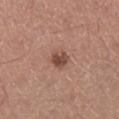<tbp_lesion>
<biopsy_status>not biopsied; imaged during a skin examination</biopsy_status>
<automated_metrics>
  <area_mm2_approx>4.5</area_mm2_approx>
  <shape_asymmetry>0.2</shape_asymmetry>
  <cielab_L>47</cielab_L>
  <cielab_a>21</cielab_a>
  <cielab_b>25</cielab_b>
  <vs_skin_darker_L>12.0</vs_skin_darker_L>
  <vs_skin_contrast_norm>8.5</vs_skin_contrast_norm>
  <border_irregularity_0_10>2.0</border_irregularity_0_10>
  <color_variation_0_10>3.0</color_variation_0_10>
  <peripheral_color_asymmetry>1.0</peripheral_color_asymmetry>
</automated_metrics>
<lighting>white-light</lighting>
<lesion_size>
  <long_diameter_mm_approx>2.5</long_diameter_mm_approx>
</lesion_size>
<site>right lower leg</site>
<image>
  <source>total-body photography crop</source>
  <field_of_view_mm>15</field_of_view_mm>
</image>
<patient>
  <sex>male</sex>
  <age_approx>40</age_approx>
</patient>
</tbp_lesion>